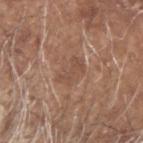This is a white-light tile.
A 15 mm crop from a total-body photograph taken for skin-cancer surveillance.
A male patient, aged around 80.
From the head or neck.
Measured at roughly 3.5 mm in maximum diameter.